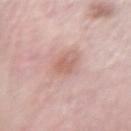The lesion's longest dimension is about 2.5 mm.
Cropped from a total-body skin-imaging series; the visible field is about 15 mm.
Imaged with white-light lighting.
The patient is a female in their mid-60s.
Located on the left forearm.